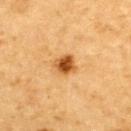notes — imaged on a skin check; not biopsied | subject — male, aged approximately 85 or older | TBP lesion metrics — an average lesion color of about L≈48 a*≈23 b*≈41 (CIELAB) and a normalized border contrast of about 10.5; a classifier nevus-likeness of about 100/100 and lesion-presence confidence of about 100/100 | lesion diameter — ≈2.5 mm | imaging modality — ~15 mm crop, total-body skin-cancer survey | lighting — cross-polarized illumination | anatomic site — the back.